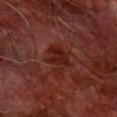Recorded during total-body skin imaging; not selected for excision or biopsy.
A male subject, roughly 80 years of age.
Cropped from a total-body skin-imaging series; the visible field is about 15 mm.
Located on the right forearm.